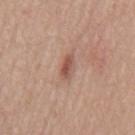automated_metrics:
  area_mm2_approx: 4.0
  eccentricity: 0.85
  shape_asymmetry: 0.15
  cielab_L: 53
  cielab_a: 22
  cielab_b: 27
  vs_skin_darker_L: 11.0
  border_irregularity_0_10: 1.5
  color_variation_0_10: 4.0
  peripheral_color_asymmetry: 1.5
image:
  source: total-body photography crop
  field_of_view_mm: 15
patient:
  sex: male
  age_approx: 65
site: mid back
lesion_size:
  long_diameter_mm_approx: 2.5